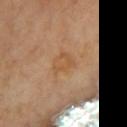The subject is female. The lesion is on the chest. A roughly 15 mm field-of-view crop from a total-body skin photograph. The total-body-photography lesion software estimated a footprint of about 6.5 mm², a shape eccentricity near 0.6, and two-axis asymmetry of about 0.25. The software also gave a normalized lesion–skin contrast near 5.5.